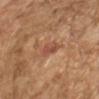biopsy_status: not biopsied; imaged during a skin examination
patient:
  sex: male
  age_approx: 60
site: arm
image:
  source: total-body photography crop
  field_of_view_mm: 15
lighting: cross-polarized
automated_metrics:
  area_mm2_approx: 4.0
  eccentricity: 0.75
  shape_asymmetry: 0.2
  border_irregularity_0_10: 2.5
  color_variation_0_10: 3.5
lesion_size:
  long_diameter_mm_approx: 2.5